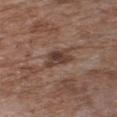biopsy_status: not biopsied; imaged during a skin examination
patient:
  sex: female
  age_approx: 75
image:
  source: total-body photography crop
  field_of_view_mm: 15
lesion_size:
  long_diameter_mm_approx: 4.0
lighting: white-light
site: chest